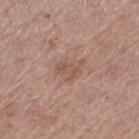Findings:
* workup — total-body-photography surveillance lesion; no biopsy
* size — ~3.5 mm (longest diameter)
* image-analysis metrics — an area of roughly 5.5 mm² and an eccentricity of roughly 0.7; a mean CIELAB color near L≈53 a*≈20 b*≈28 and about 7 CIELAB-L* units darker than the surrounding skin
* anatomic site — the left thigh
* image source — ~15 mm tile from a whole-body skin photo
* patient — female, approximately 60 years of age